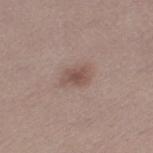This lesion was catalogued during total-body skin photography and was not selected for biopsy.
A 15 mm close-up extracted from a 3D total-body photography capture.
The subject is a female about 30 years old.
On the left thigh.